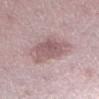follow-up — total-body-photography surveillance lesion; no biopsy | patient — female, aged around 45 | body site — the left lower leg | lesion diameter — about 6 mm | image-analysis metrics — an average lesion color of about L≈57 a*≈19 b*≈17 (CIELAB) and a normalized border contrast of about 6.5; an automated nevus-likeness rating near 15 out of 100 and a lesion-detection confidence of about 90/100 | tile lighting — white-light | imaging modality — ~15 mm crop, total-body skin-cancer survey.